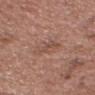Part of a total-body skin-imaging series; this lesion was reviewed on a skin check and was not flagged for biopsy. This is a white-light tile. Cropped from a total-body skin-imaging series; the visible field is about 15 mm. About 4 mm across. The lesion is on the head or neck. A male subject roughly 55 years of age.